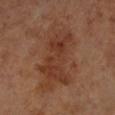follow-up = total-body-photography surveillance lesion; no biopsy | lighting = cross-polarized illumination | image-analysis metrics = a mean CIELAB color near L≈37 a*≈21 b*≈29, a lesion–skin lightness drop of about 7, and a normalized border contrast of about 6.5; a classifier nevus-likeness of about 5/100 and a detector confidence of about 100 out of 100 that the crop contains a lesion | lesion diameter = about 9 mm | imaging modality = ~15 mm crop, total-body skin-cancer survey | patient = female, approximately 65 years of age | site = the left forearm.